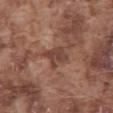No biopsy was performed on this lesion — it was imaged during a full skin examination and was not determined to be concerning. The lesion is located on the abdomen. The patient is a male aged 73–77. Cropped from a total-body skin-imaging series; the visible field is about 15 mm.